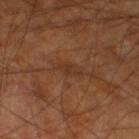workup — total-body-photography surveillance lesion; no biopsy | body site — the left lower leg | automated lesion analysis — a mean CIELAB color near L≈33 a*≈21 b*≈30, roughly 5 lightness units darker than nearby skin, and a lesion-to-skin contrast of about 5.5 (normalized; higher = more distinct); a border-irregularity index near 4/10, internal color variation of about 0.5 on a 0–10 scale, and peripheral color asymmetry of about 0 | tile lighting — cross-polarized | image — 15 mm crop, total-body photography | subject — male, aged approximately 60 | diameter — ≈3 mm.